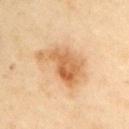About 6 mm across.
A male subject, aged 53–57.
Captured under cross-polarized illumination.
Located on the left upper arm.
A 15 mm crop from a total-body photograph taken for skin-cancer surveillance.